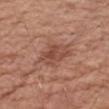Clinical impression:
The lesion was photographed on a routine skin check and not biopsied; there is no pathology result.
Clinical summary:
The lesion-visualizer software estimated a mean CIELAB color near L≈48 a*≈23 b*≈29. The analysis additionally found an automated nevus-likeness rating near 10 out of 100 and lesion-presence confidence of about 100/100. On the right upper arm. A male subject aged 58 to 62. The tile uses white-light illumination. A 15 mm close-up extracted from a 3D total-body photography capture. The recorded lesion diameter is about 4.5 mm.